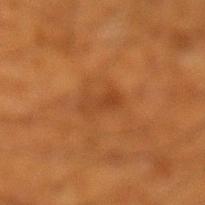Case summary:
• image-analysis metrics: a border-irregularity index near 4/10, a color-variation rating of about 3/10, and a peripheral color-asymmetry measure near 1
• acquisition: 15 mm crop, total-body photography
• patient: male, roughly 60 years of age
• lighting: cross-polarized
• size: ≈3.5 mm
• body site: the left lower leg